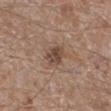Recorded during total-body skin imaging; not selected for excision or biopsy.
The recorded lesion diameter is about 3 mm.
A 15 mm close-up tile from a total-body photography series done for melanoma screening.
The subject is a male about 65 years old.
The lesion is on the right lower leg.